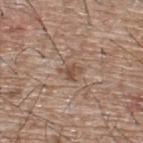Context: The tile uses white-light illumination. A male subject, aged 63–67. The lesion's longest dimension is about 2.5 mm. The total-body-photography lesion software estimated internal color variation of about 1 on a 0–10 scale. A 15 mm close-up tile from a total-body photography series done for melanoma screening. The lesion is located on the upper back.